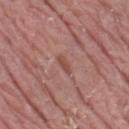subject: male, approximately 40 years of age; site: the lower back; image: ~15 mm tile from a whole-body skin photo.